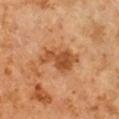{
  "biopsy_status": "not biopsied; imaged during a skin examination",
  "lesion_size": {
    "long_diameter_mm_approx": 5.0
  },
  "lighting": "cross-polarized",
  "image": {
    "source": "total-body photography crop",
    "field_of_view_mm": 15
  },
  "automated_metrics": {
    "area_mm2_approx": 11.0,
    "eccentricity": 0.85,
    "color_variation_0_10": 3.5,
    "peripheral_color_asymmetry": 1.5
  },
  "patient": {
    "sex": "male",
    "age_approx": 60
  },
  "site": "arm"
}